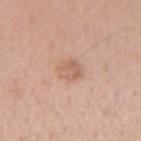Imaged during a routine full-body skin examination; the lesion was not biopsied and no histopathology is available. The lesion is on the right upper arm. The lesion-visualizer software estimated an area of roughly 6 mm², a shape eccentricity near 0.55, and a symmetry-axis asymmetry near 0.15. And it measured a mean CIELAB color near L≈62 a*≈20 b*≈30, a lesion–skin lightness drop of about 8, and a normalized lesion–skin contrast near 5.5. The analysis additionally found a border-irregularity rating of about 2/10, a within-lesion color-variation index near 3/10, and peripheral color asymmetry of about 1. The analysis additionally found a nevus-likeness score of about 10/100 and lesion-presence confidence of about 100/100. The recorded lesion diameter is about 3 mm. A roughly 15 mm field-of-view crop from a total-body skin photograph. A female patient aged 38 to 42. Captured under white-light illumination.